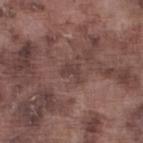The lesion was tiled from a total-body skin photograph and was not biopsied.
Longest diameter approximately 2.5 mm.
The patient is a male aged 73–77.
Located on the leg.
This is a white-light tile.
Cropped from a total-body skin-imaging series; the visible field is about 15 mm.